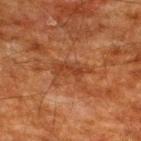biopsy status=no biopsy performed (imaged during a skin exam)
imaging modality=total-body-photography crop, ~15 mm field of view
site=the upper back
illumination=cross-polarized
subject=male, aged 58 to 62